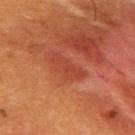<record>
  <biopsy_status>not biopsied; imaged during a skin examination</biopsy_status>
  <lesion_size>
    <long_diameter_mm_approx>4.5</long_diameter_mm_approx>
  </lesion_size>
  <lighting>cross-polarized</lighting>
  <patient>
    <sex>male</sex>
    <age_approx>50</age_approx>
  </patient>
  <image>
    <source>total-body photography crop</source>
    <field_of_view_mm>15</field_of_view_mm>
  </image>
  <site>back</site>
  <automated_metrics>
    <area_mm2_approx>6.5</area_mm2_approx>
    <eccentricity>0.95</eccentricity>
    <shape_asymmetry>0.35</shape_asymmetry>
    <vs_skin_darker_L>5.0</vs_skin_darker_L>
    <vs_skin_contrast_norm>5.0</vs_skin_contrast_norm>
    <border_irregularity_0_10>4.5</border_irregularity_0_10>
  </automated_metrics>
</record>